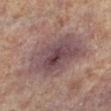{"biopsy_status": "not biopsied; imaged during a skin examination", "automated_metrics": {"cielab_L": 41, "cielab_a": 16, "cielab_b": 15, "vs_skin_contrast_norm": 9.0, "border_irregularity_0_10": 3.0, "color_variation_0_10": 5.5, "lesion_detection_confidence_0_100": 95}, "site": "left lower leg", "image": {"source": "total-body photography crop", "field_of_view_mm": 15}, "lesion_size": {"long_diameter_mm_approx": 8.5}, "lighting": "cross-polarized", "patient": {"sex": "male", "age_approx": 65}}